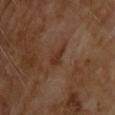– notes: imaged on a skin check; not biopsied
– image-analysis metrics: an area of roughly 3 mm², an eccentricity of roughly 0.8, and a symmetry-axis asymmetry near 0.35
– patient: male, approximately 70 years of age
– lesion size: ≈2.5 mm
– site: the back
– image: ~15 mm tile from a whole-body skin photo